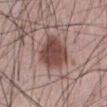  patient:
    sex: male
    age_approx: 45
  automated_metrics:
    border_irregularity_0_10: 2.5
    color_variation_0_10: 4.0
    nevus_likeness_0_100: 95
    lesion_detection_confidence_0_100: 100
  site: abdomen
  image:
    source: total-body photography crop
    field_of_view_mm: 15